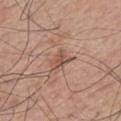Q: What are the patient's age and sex?
A: male, aged approximately 70
Q: What is the lesion's diameter?
A: ~3 mm (longest diameter)
Q: How was the tile lit?
A: white-light
Q: What kind of image is this?
A: 15 mm crop, total-body photography
Q: What is the anatomic site?
A: the back